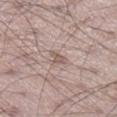This lesion was catalogued during total-body skin photography and was not selected for biopsy. The lesion-visualizer software estimated an average lesion color of about L≈56 a*≈16 b*≈22 (CIELAB), about 9 CIELAB-L* units darker than the surrounding skin, and a normalized border contrast of about 6.5. The software also gave a border-irregularity rating of about 4/10, a within-lesion color-variation index near 0.5/10, and a peripheral color-asymmetry measure near 0.5. It also reported a classifier nevus-likeness of about 0/100 and a detector confidence of about 60 out of 100 that the crop contains a lesion. A male subject aged approximately 60. Located on the leg. The recorded lesion diameter is about 2.5 mm. A close-up tile cropped from a whole-body skin photograph, about 15 mm across. The tile uses white-light illumination.